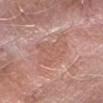Recorded during total-body skin imaging; not selected for excision or biopsy.
A male subject, roughly 65 years of age.
A 15 mm crop from a total-body photograph taken for skin-cancer surveillance.
The lesion-visualizer software estimated a lesion color around L≈58 a*≈22 b*≈26 in CIELAB. And it measured an automated nevus-likeness rating near 0 out of 100 and lesion-presence confidence of about 80/100.
This is a white-light tile.
Approximately 6.5 mm at its widest.
Located on the right forearm.